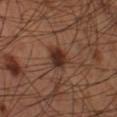notes — imaged on a skin check; not biopsied
anatomic site — the leg
image-analysis metrics — a footprint of about 6.5 mm², a shape eccentricity near 0.6, and a shape-asymmetry score of about 0.25 (0 = symmetric); a border-irregularity index near 2/10 and radial color variation of about 1.5
patient — male, roughly 60 years of age
image source — 15 mm crop, total-body photography
illumination — cross-polarized illumination